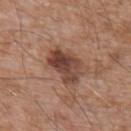Q: Was a biopsy performed?
A: total-body-photography surveillance lesion; no biopsy
Q: Lesion location?
A: the chest
Q: How was the tile lit?
A: white-light
Q: Who is the patient?
A: male, in their 60s
Q: How was this image acquired?
A: ~15 mm tile from a whole-body skin photo
Q: How large is the lesion?
A: about 5 mm
Q: What did automated image analysis measure?
A: an area of roughly 12 mm², a shape eccentricity near 0.75, and two-axis asymmetry of about 0.3; an average lesion color of about L≈43 a*≈21 b*≈26 (CIELAB) and a normalized border contrast of about 10; a nevus-likeness score of about 35/100 and a lesion-detection confidence of about 100/100